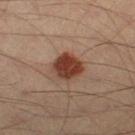From the leg. Captured under cross-polarized illumination. A 15 mm crop from a total-body photograph taken for skin-cancer surveillance. The lesion's longest dimension is about 3.5 mm. The subject is a male aged 38 to 42.